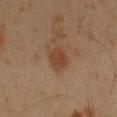{"biopsy_status": "not biopsied; imaged during a skin examination", "site": "left forearm", "image": {"source": "total-body photography crop", "field_of_view_mm": 15}, "patient": {"sex": "male", "age_approx": 45}}